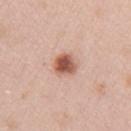Assessment: No biopsy was performed on this lesion — it was imaged during a full skin examination and was not determined to be concerning.